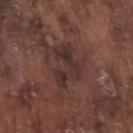Clinical impression:
The lesion was photographed on a routine skin check and not biopsied; there is no pathology result.
Context:
A 15 mm close-up tile from a total-body photography series done for melanoma screening. The tile uses white-light illumination. The patient is a male in their mid- to late 70s. About 4.5 mm across. From the left upper arm.